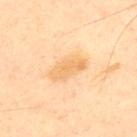{"biopsy_status": "not biopsied; imaged during a skin examination", "lesion_size": {"long_diameter_mm_approx": 4.5}, "image": {"source": "total-body photography crop", "field_of_view_mm": 15}, "automated_metrics": {"area_mm2_approx": 9.5, "eccentricity": 0.85, "shape_asymmetry": 0.2, "cielab_L": 75, "cielab_a": 19, "cielab_b": 44, "vs_skin_darker_L": 8.0, "vs_skin_contrast_norm": 6.0, "nevus_likeness_0_100": 0, "lesion_detection_confidence_0_100": 100}, "patient": {"sex": "male", "age_approx": 45}, "lighting": "cross-polarized", "site": "upper back"}